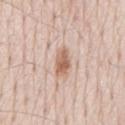{"biopsy_status": "not biopsied; imaged during a skin examination", "site": "mid back", "patient": {"sex": "male", "age_approx": 80}, "image": {"source": "total-body photography crop", "field_of_view_mm": 15}}